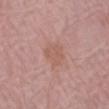No biopsy was performed on this lesion — it was imaged during a full skin examination and was not determined to be concerning. From the right forearm. Cropped from a total-body skin-imaging series; the visible field is about 15 mm. Longest diameter approximately 3 mm. A female subject about 65 years old.